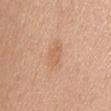biopsy status = total-body-photography surveillance lesion; no biopsy | image = ~15 mm crop, total-body skin-cancer survey | body site = the abdomen | patient = female, approximately 65 years of age.